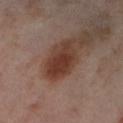Imaged during a routine full-body skin examination; the lesion was not biopsied and no histopathology is available. A region of skin cropped from a whole-body photographic capture, roughly 15 mm wide. The patient is a female approximately 55 years of age. From the left lower leg.